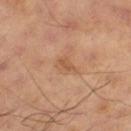workup: no biopsy performed (imaged during a skin exam)
anatomic site: the right thigh
imaging modality: 15 mm crop, total-body photography